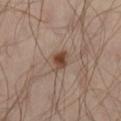Findings:
- follow-up — total-body-photography surveillance lesion; no biopsy
- patient — male, in their mid- to late 40s
- image source — total-body-photography crop, ~15 mm field of view
- illumination — cross-polarized illumination
- site — the leg